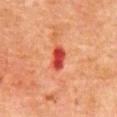Q: Is there a histopathology result?
A: catalogued during a skin exam; not biopsied
Q: What is the imaging modality?
A: 15 mm crop, total-body photography
Q: What is the anatomic site?
A: the abdomen
Q: How large is the lesion?
A: ≈3 mm
Q: Automated lesion metrics?
A: a footprint of about 4.5 mm², an eccentricity of roughly 0.85, and a shape-asymmetry score of about 0.2 (0 = symmetric)
Q: What lighting was used for the tile?
A: cross-polarized illumination
Q: Who is the patient?
A: male, approximately 80 years of age